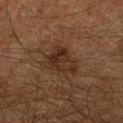Impression:
Imaged during a routine full-body skin examination; the lesion was not biopsied and no histopathology is available.
Image and clinical context:
From the right lower leg. The patient is a male in their 60s. Measured at roughly 4.5 mm in maximum diameter. A close-up tile cropped from a whole-body skin photograph, about 15 mm across. Automated image analysis of the tile measured an area of roughly 10 mm², an outline eccentricity of about 0.75 (0 = round, 1 = elongated), and a shape-asymmetry score of about 0.35 (0 = symmetric). The software also gave an average lesion color of about L≈22 a*≈14 b*≈22 (CIELAB), about 6 CIELAB-L* units darker than the surrounding skin, and a lesion-to-skin contrast of about 7.5 (normalized; higher = more distinct). And it measured a border-irregularity rating of about 4/10, internal color variation of about 4 on a 0–10 scale, and radial color variation of about 1.5. This is a cross-polarized tile.